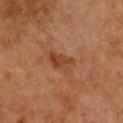follow-up — total-body-photography surveillance lesion; no biopsy | patient — female, roughly 60 years of age | site — the chest | image source — 15 mm crop, total-body photography.